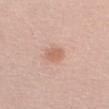Recorded during total-body skin imaging; not selected for excision or biopsy. A female patient, aged approximately 30. The recorded lesion diameter is about 3 mm. The tile uses white-light illumination. A 15 mm close-up extracted from a 3D total-body photography capture. The lesion is on the arm. Automated image analysis of the tile measured a lesion area of about 4.5 mm².